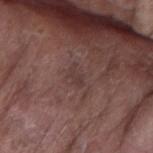The lesion was photographed on a routine skin check and not biopsied; there is no pathology result.
Imaged with white-light lighting.
Located on the right forearm.
A close-up tile cropped from a whole-body skin photograph, about 15 mm across.
A male subject, aged around 75.
An algorithmic analysis of the crop reported a footprint of about 3 mm² and two-axis asymmetry of about 0.4. And it measured a mean CIELAB color near L≈37 a*≈17 b*≈18, a lesion–skin lightness drop of about 5, and a normalized border contrast of about 5. And it measured a border-irregularity rating of about 4.5/10.
About 2.5 mm across.